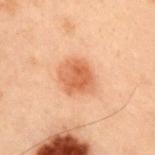notes: catalogued during a skin exam; not biopsied | subject: male, about 55 years old | image-analysis metrics: a shape eccentricity near 0.5 and a shape-asymmetry score of about 0.15 (0 = symmetric) | location: the right upper arm | imaging modality: ~15 mm tile from a whole-body skin photo | lighting: cross-polarized illumination | lesion size: about 3.5 mm.